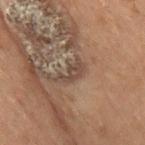| field | value |
|---|---|
| follow-up | no biopsy performed (imaged during a skin exam) |
| patient | male, aged 68 to 72 |
| diameter | about 3 mm |
| imaging modality | 15 mm crop, total-body photography |
| automated metrics | roughly 6 lightness units darker than nearby skin and a normalized lesion–skin contrast near 6.5; a border-irregularity rating of about 4.5/10, a within-lesion color-variation index near 1.5/10, and peripheral color asymmetry of about 0.5; a classifier nevus-likeness of about 0/100 and a detector confidence of about 80 out of 100 that the crop contains a lesion |
| lighting | cross-polarized illumination |
| site | the right thigh |